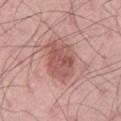Captured during whole-body skin photography for melanoma surveillance; the lesion was not biopsied. A male patient, aged 43 to 47. Longest diameter approximately 5.5 mm. Automated tile analysis of the lesion measured a lesion–skin lightness drop of about 11. The software also gave border irregularity of about 2.5 on a 0–10 scale, internal color variation of about 4 on a 0–10 scale, and peripheral color asymmetry of about 1.5. Cropped from a total-body skin-imaging series; the visible field is about 15 mm. From the right thigh. The tile uses white-light illumination.